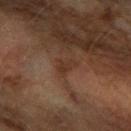Imaged during a routine full-body skin examination; the lesion was not biopsied and no histopathology is available.
A female patient, approximately 60 years of age.
The lesion-visualizer software estimated a lesion color around L≈29 a*≈16 b*≈24 in CIELAB and a lesion-to-skin contrast of about 5.5 (normalized; higher = more distinct). It also reported lesion-presence confidence of about 80/100.
On the left forearm.
Imaged with cross-polarized lighting.
Approximately 3 mm at its widest.
This image is a 15 mm lesion crop taken from a total-body photograph.